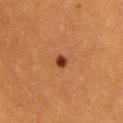Recorded during total-body skin imaging; not selected for excision or biopsy.
Captured under cross-polarized illumination.
Located on the lower back.
A 15 mm close-up extracted from a 3D total-body photography capture.
The subject is a female in their 30s.
About 2 mm across.